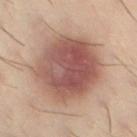biopsy status: no biopsy performed (imaged during a skin exam)
automated lesion analysis: a lesion area of about 40 mm² and a symmetry-axis asymmetry near 0.1; a lesion color around L≈41 a*≈18 b*≈20 in CIELAB, roughly 11 lightness units darker than nearby skin, and a normalized lesion–skin contrast near 9.5; border irregularity of about 1 on a 0–10 scale, internal color variation of about 5.5 on a 0–10 scale, and radial color variation of about 2
illumination: cross-polarized illumination
image: 15 mm crop, total-body photography
patient: male, aged around 30
body site: the left thigh
size: ~7.5 mm (longest diameter)